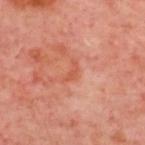This lesion was catalogued during total-body skin photography and was not selected for biopsy. The lesion is on the upper back. A 15 mm close-up extracted from a 3D total-body photography capture. The patient is aged 53 to 57. The tile uses cross-polarized illumination.